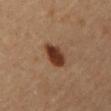Q: Was a biopsy performed?
A: total-body-photography surveillance lesion; no biopsy
Q: Lesion size?
A: about 3.5 mm
Q: Lesion location?
A: the chest
Q: How was this image acquired?
A: total-body-photography crop, ~15 mm field of view
Q: How was the tile lit?
A: cross-polarized illumination
Q: Patient demographics?
A: male, in their mid- to late 60s